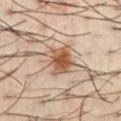{"biopsy_status": "not biopsied; imaged during a skin examination", "automated_metrics": {"border_irregularity_0_10": 3.0, "peripheral_color_asymmetry": 1.5, "lesion_detection_confidence_0_100": 100}, "patient": {"sex": "male", "age_approx": 40}, "lesion_size": {"long_diameter_mm_approx": 3.5}, "site": "chest", "image": {"source": "total-body photography crop", "field_of_view_mm": 15}, "lighting": "cross-polarized"}